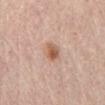Case summary:
* workup — no biopsy performed (imaged during a skin exam)
* image source — ~15 mm tile from a whole-body skin photo
* patient — female, in their mid-60s
* site — the abdomen